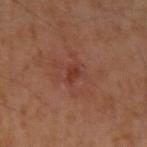site: the arm | patient: male, aged 48 to 52 | image: total-body-photography crop, ~15 mm field of view | histopathology: a seborrheic keratosis — a benign lesion.